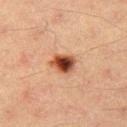{
  "biopsy_status": "not biopsied; imaged during a skin examination",
  "automated_metrics": {
    "border_irregularity_0_10": 2.0,
    "peripheral_color_asymmetry": 2.5,
    "nevus_likeness_0_100": 100
  },
  "lesion_size": {
    "long_diameter_mm_approx": 3.0
  },
  "lighting": "cross-polarized",
  "image": {
    "source": "total-body photography crop",
    "field_of_view_mm": 15
  },
  "patient": {
    "sex": "male",
    "age_approx": 40
  },
  "site": "left thigh"
}